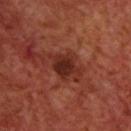Recorded during total-body skin imaging; not selected for excision or biopsy. A 15 mm crop from a total-body photograph taken for skin-cancer surveillance. Located on the upper back. A male patient, aged around 70. Imaged with cross-polarized lighting. The lesion's longest dimension is about 3 mm.